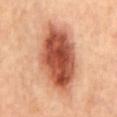workup = total-body-photography surveillance lesion; no biopsy
lesion diameter = about 10 mm
illumination = cross-polarized
image source = total-body-photography crop, ~15 mm field of view
body site = the mid back